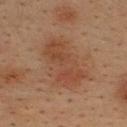Assessment: The lesion was tiled from a total-body skin photograph and was not biopsied. Background: From the upper back. The lesion's longest dimension is about 7 mm. A male subject, aged 33–37. A 15 mm close-up extracted from a 3D total-body photography capture. This is a cross-polarized tile.